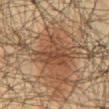Recorded during total-body skin imaging; not selected for excision or biopsy. Located on the mid back. A 15 mm close-up tile from a total-body photography series done for melanoma screening. A male subject aged around 50. The lesion's longest dimension is about 5.5 mm.